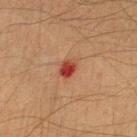Findings:
* follow-up: total-body-photography surveillance lesion; no biopsy
* acquisition: 15 mm crop, total-body photography
* diameter: ~2.5 mm (longest diameter)
* TBP lesion metrics: a classifier nevus-likeness of about 0/100 and lesion-presence confidence of about 100/100
* patient: male, aged approximately 50
* site: the left lower leg
* illumination: cross-polarized